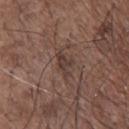biopsy status=no biopsy performed (imaged during a skin exam)
illumination=white-light
image-analysis metrics=a footprint of about 4 mm² and an eccentricity of roughly 0.85; a nevus-likeness score of about 0/100 and a lesion-detection confidence of about 80/100
patient=male, approximately 70 years of age
location=the front of the torso
diameter=about 3 mm
imaging modality=~15 mm tile from a whole-body skin photo